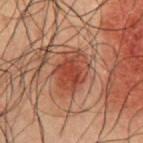{
  "lighting": "cross-polarized",
  "site": "abdomen",
  "patient": {
    "sex": "male",
    "age_approx": 50
  },
  "lesion_size": {
    "long_diameter_mm_approx": 4.5
  },
  "image": {
    "source": "total-body photography crop",
    "field_of_view_mm": 15
  }
}